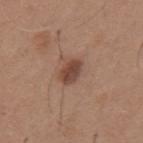Q: Was a biopsy performed?
A: imaged on a skin check; not biopsied
Q: What lighting was used for the tile?
A: white-light illumination
Q: Automated lesion metrics?
A: roughly 12 lightness units darker than nearby skin; border irregularity of about 1.5 on a 0–10 scale and a color-variation rating of about 2.5/10; lesion-presence confidence of about 100/100
Q: Lesion location?
A: the mid back
Q: How was this image acquired?
A: total-body-photography crop, ~15 mm field of view
Q: Lesion size?
A: ≈3 mm
Q: Patient demographics?
A: male, aged 63 to 67